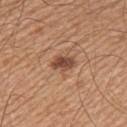{"biopsy_status": "not biopsied; imaged during a skin examination", "patient": {"sex": "male", "age_approx": 65}, "image": {"source": "total-body photography crop", "field_of_view_mm": 15}, "site": "left upper arm", "lesion_size": {"long_diameter_mm_approx": 3.0}, "lighting": "white-light"}